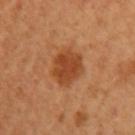A female subject, aged 53–57. The lesion is on the arm. Longest diameter approximately 4 mm. A roughly 15 mm field-of-view crop from a total-body skin photograph. The tile uses cross-polarized illumination.